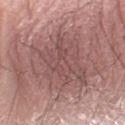Impression:
This lesion was catalogued during total-body skin photography and was not selected for biopsy.
Context:
A close-up tile cropped from a whole-body skin photograph, about 15 mm across. The total-body-photography lesion software estimated a lesion area of about 44 mm², a shape eccentricity near 0.6, and a shape-asymmetry score of about 0.2 (0 = symmetric). And it measured an average lesion color of about L≈52 a*≈21 b*≈20 (CIELAB), roughly 8 lightness units darker than nearby skin, and a normalized border contrast of about 6. The patient is a male in their mid-70s. Located on the left forearm. Imaged with white-light lighting.